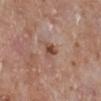Part of a total-body skin-imaging series; this lesion was reviewed on a skin check and was not flagged for biopsy.
This is a white-light tile.
From the left lower leg.
Cropped from a total-body skin-imaging series; the visible field is about 15 mm.
A female patient in their mid-70s.